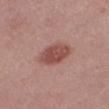Part of a total-body skin-imaging series; this lesion was reviewed on a skin check and was not flagged for biopsy.